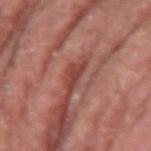biopsy status — imaged on a skin check; not biopsied
size — ~3.5 mm (longest diameter)
acquisition — 15 mm crop, total-body photography
anatomic site — the left upper arm
subject — male, about 80 years old